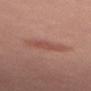<record>
  <biopsy_status>not biopsied; imaged during a skin examination</biopsy_status>
  <site>left thigh</site>
  <patient>
    <sex>female</sex>
    <age_approx>40</age_approx>
  </patient>
  <lighting>white-light</lighting>
  <image>
    <source>total-body photography crop</source>
    <field_of_view_mm>15</field_of_view_mm>
  </image>
</record>